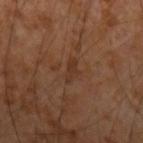Recorded during total-body skin imaging; not selected for excision or biopsy. On the left forearm. A 15 mm close-up extracted from a 3D total-body photography capture. A male subject, aged 53 to 57. Approximately 3 mm at its widest.